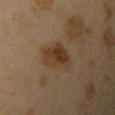Findings:
• follow-up: catalogued during a skin exam; not biopsied
• diameter: ~4.5 mm (longest diameter)
• patient: female, aged 38 to 42
• anatomic site: the left upper arm
• image: ~15 mm crop, total-body skin-cancer survey
• TBP lesion metrics: a lesion area of about 11 mm², an eccentricity of roughly 0.75, and two-axis asymmetry of about 0.25; an average lesion color of about L≈29 a*≈12 b*≈25 (CIELAB) and a normalized lesion–skin contrast near 8; a border-irregularity index near 2.5/10, internal color variation of about 4.5 on a 0–10 scale, and a peripheral color-asymmetry measure near 1.5
• lighting: cross-polarized illumination